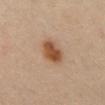biopsy status — total-body-photography surveillance lesion; no biopsy | patient — male, in their mid- to late 60s | image source — ~15 mm crop, total-body skin-cancer survey | location — the abdomen.